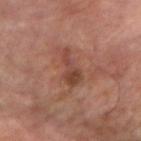Acquisition and patient details:
Automated tile analysis of the lesion measured a lesion area of about 6 mm². The software also gave a classifier nevus-likeness of about 0/100 and a lesion-detection confidence of about 100/100. A lesion tile, about 15 mm wide, cut from a 3D total-body photograph. The recorded lesion diameter is about 4.5 mm. This is a cross-polarized tile. The lesion is on the arm. The subject is a male aged 63–67.